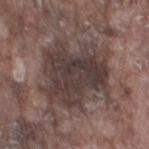biopsy_status: not biopsied; imaged during a skin examination
patient:
  sex: male
  age_approx: 75
site: left thigh
lesion_size:
  long_diameter_mm_approx: 8.0
image:
  source: total-body photography crop
  field_of_view_mm: 15
lighting: white-light